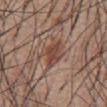Findings:
* biopsy status: total-body-photography surveillance lesion; no biopsy
* illumination: white-light illumination
* anatomic site: the abdomen
* image source: ~15 mm crop, total-body skin-cancer survey
* subject: male, about 55 years old